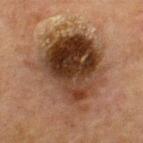Notes:
- workup — catalogued during a skin exam; not biopsied
- image source — ~15 mm tile from a whole-body skin photo
- tile lighting — cross-polarized illumination
- image-analysis metrics — about 13 CIELAB-L* units darker than the surrounding skin and a normalized border contrast of about 12; a border-irregularity rating of about 5/10, a within-lesion color-variation index near 9.5/10, and a peripheral color-asymmetry measure near 4; a nevus-likeness score of about 15/100 and lesion-presence confidence of about 100/100
- patient — male, in their mid- to late 60s
- body site — the front of the torso